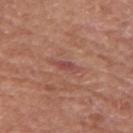follow-up: no biopsy performed (imaged during a skin exam)
image: total-body-photography crop, ~15 mm field of view
subject: male, aged around 80
tile lighting: white-light illumination
anatomic site: the left forearm